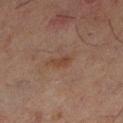follow-up: imaged on a skin check; not biopsied
automated lesion analysis: an outline eccentricity of about 0.85 (0 = round, 1 = elongated); a border-irregularity rating of about 3/10, a color-variation rating of about 1/10, and peripheral color asymmetry of about 0.5; lesion-presence confidence of about 100/100
anatomic site: the left lower leg
lesion size: about 2.5 mm
image source: ~15 mm crop, total-body skin-cancer survey
lighting: cross-polarized
subject: male, roughly 45 years of age